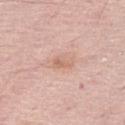A 15 mm crop from a total-body photograph taken for skin-cancer surveillance.
The total-body-photography lesion software estimated a classifier nevus-likeness of about 0/100 and a lesion-detection confidence of about 100/100.
Captured under white-light illumination.
Measured at roughly 2.5 mm in maximum diameter.
The lesion is on the leg.
A female patient, approximately 65 years of age.